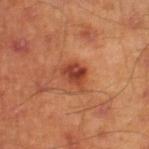Notes:
* follow-up · total-body-photography surveillance lesion; no biopsy
* size · ~3.5 mm (longest diameter)
* illumination · cross-polarized illumination
* body site · the right lower leg
* image · 15 mm crop, total-body photography
* patient · male, aged 43–47
* automated lesion analysis · an area of roughly 5.5 mm², a shape eccentricity near 0.7, and a shape-asymmetry score of about 0.35 (0 = symmetric); a mean CIELAB color near L≈44 a*≈31 b*≈34 and a normalized lesion–skin contrast near 9; a border-irregularity rating of about 3/10, internal color variation of about 4 on a 0–10 scale, and a peripheral color-asymmetry measure near 1.5